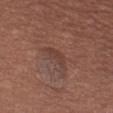Part of a total-body skin-imaging series; this lesion was reviewed on a skin check and was not flagged for biopsy. Located on the left thigh. Measured at roughly 3.5 mm in maximum diameter. A female patient, in their mid-50s. A lesion tile, about 15 mm wide, cut from a 3D total-body photograph.